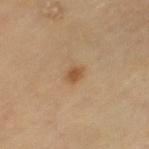| key | value |
|---|---|
| follow-up | imaged on a skin check; not biopsied |
| body site | the left thigh |
| image | ~15 mm tile from a whole-body skin photo |
| automated lesion analysis | border irregularity of about 1.5 on a 0–10 scale, a within-lesion color-variation index near 1.5/10, and a peripheral color-asymmetry measure near 0.5; an automated nevus-likeness rating near 85 out of 100 and a lesion-detection confidence of about 100/100 |
| lighting | cross-polarized |
| subject | female, aged 68 to 72 |
| lesion diameter | ≈2 mm |